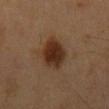Findings:
- notes: imaged on a skin check; not biopsied
- diameter: ≈5.5 mm
- acquisition: 15 mm crop, total-body photography
- site: the mid back
- subject: male, approximately 60 years of age
- automated metrics: an area of roughly 12 mm², an eccentricity of roughly 0.8, and a symmetry-axis asymmetry near 0.1; border irregularity of about 1.5 on a 0–10 scale and radial color variation of about 1; a classifier nevus-likeness of about 100/100